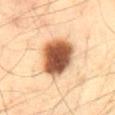Part of a total-body skin-imaging series; this lesion was reviewed on a skin check and was not flagged for biopsy. Captured under cross-polarized illumination. A male subject about 40 years old. Measured at roughly 5.5 mm in maximum diameter. The lesion is on the back. A 15 mm close-up extracted from a 3D total-body photography capture.